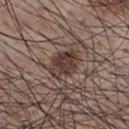Imaged during a routine full-body skin examination; the lesion was not biopsied and no histopathology is available. A male subject, in their mid-50s. A region of skin cropped from a whole-body photographic capture, roughly 15 mm wide. From the chest. Captured under white-light illumination. Longest diameter approximately 4 mm.